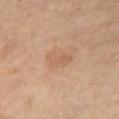Clinical impression:
The lesion was tiled from a total-body skin photograph and was not biopsied.
Acquisition and patient details:
The tile uses cross-polarized illumination. A 15 mm close-up tile from a total-body photography series done for melanoma screening. The lesion is on the right thigh. The lesion-visualizer software estimated an area of roughly 5 mm² and an eccentricity of roughly 0.85. The software also gave a mean CIELAB color near L≈61 a*≈19 b*≈34 and a normalized lesion–skin contrast near 4.5. And it measured a border-irregularity index near 3/10 and a color-variation rating of about 2.5/10. It also reported a nevus-likeness score of about 10/100 and a lesion-detection confidence of about 100/100. A female subject in their mid-50s.